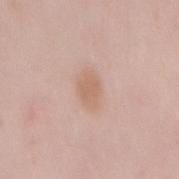The lesion was photographed on a routine skin check and not biopsied; there is no pathology result. Measured at roughly 4 mm in maximum diameter. A 15 mm crop from a total-body photograph taken for skin-cancer surveillance. The lesion is located on the mid back. A female patient approximately 40 years of age. Captured under white-light illumination.